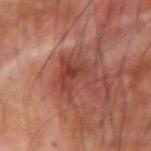Case summary:
• image — ~15 mm tile from a whole-body skin photo
• location — the arm
• lesion size — ~5 mm (longest diameter)
• patient — male, approximately 70 years of age